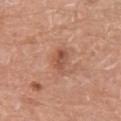Captured during whole-body skin photography for melanoma surveillance; the lesion was not biopsied. Located on the leg. This is a white-light tile. A 15 mm crop from a total-body photograph taken for skin-cancer surveillance. The lesion's longest dimension is about 3.5 mm. A female subject aged 73 to 77.